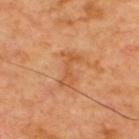Q: Is there a histopathology result?
A: imaged on a skin check; not biopsied
Q: Automated lesion metrics?
A: an automated nevus-likeness rating near 0 out of 100
Q: Patient demographics?
A: in their mid-60s
Q: What is the imaging modality?
A: ~15 mm tile from a whole-body skin photo
Q: What is the anatomic site?
A: the back